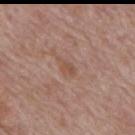Case summary:
• automated metrics: a footprint of about 3 mm² and a shape eccentricity near 0.85; roughly 6 lightness units darker than nearby skin and a normalized border contrast of about 5.5; a border-irregularity index near 3.5/10 and peripheral color asymmetry of about 0
• location: the mid back
• imaging modality: total-body-photography crop, ~15 mm field of view
• lighting: white-light
• subject: male, aged approximately 70